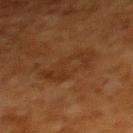workup = no biopsy performed (imaged during a skin exam); location = the upper back; diameter = ≈6.5 mm; imaging modality = 15 mm crop, total-body photography; subject = female, aged around 50.